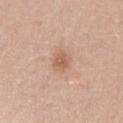Acquisition and patient details: The recorded lesion diameter is about 2.5 mm. Imaged with white-light lighting. The lesion-visualizer software estimated a nevus-likeness score of about 70/100. The subject is a female aged approximately 40. A 15 mm crop from a total-body photograph taken for skin-cancer surveillance. Located on the left upper arm.